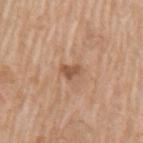Clinical impression: Recorded during total-body skin imaging; not selected for excision or biopsy. Image and clinical context: This is a white-light tile. Longest diameter approximately 2.5 mm. The lesion is on the arm. The patient is a male approximately 60 years of age. A close-up tile cropped from a whole-body skin photograph, about 15 mm across.